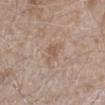Q: Is there a histopathology result?
A: catalogued during a skin exam; not biopsied
Q: What is the lesion's diameter?
A: ≈3 mm
Q: Illumination type?
A: white-light
Q: What are the patient's age and sex?
A: male, aged 43–47
Q: What kind of image is this?
A: total-body-photography crop, ~15 mm field of view
Q: Automated lesion metrics?
A: a lesion area of about 4.5 mm², an outline eccentricity of about 0.7 (0 = round, 1 = elongated), and two-axis asymmetry of about 0.4; a lesion color around L≈56 a*≈15 b*≈27 in CIELAB, roughly 7 lightness units darker than nearby skin, and a lesion-to-skin contrast of about 5.5 (normalized; higher = more distinct); a nevus-likeness score of about 0/100
Q: Where on the body is the lesion?
A: the leg